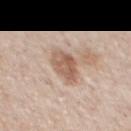Q: Was a biopsy performed?
A: imaged on a skin check; not biopsied
Q: What is the lesion's diameter?
A: ≈4.5 mm
Q: Where on the body is the lesion?
A: the chest
Q: Patient demographics?
A: male, in their mid-50s
Q: What is the imaging modality?
A: ~15 mm tile from a whole-body skin photo
Q: What lighting was used for the tile?
A: white-light
Q: What did automated image analysis measure?
A: an average lesion color of about L≈59 a*≈18 b*≈28 (CIELAB), a lesion–skin lightness drop of about 12, and a lesion-to-skin contrast of about 8 (normalized; higher = more distinct); border irregularity of about 2.5 on a 0–10 scale, a color-variation rating of about 4.5/10, and peripheral color asymmetry of about 1.5; an automated nevus-likeness rating near 60 out of 100